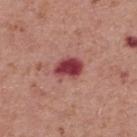Clinical summary: The lesion is located on the mid back. Automated image analysis of the tile measured a shape-asymmetry score of about 0.2 (0 = symmetric). About 3.5 mm across. The tile uses white-light illumination. Cropped from a total-body skin-imaging series; the visible field is about 15 mm. A male subject about 70 years old.